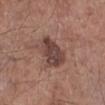  biopsy_status: not biopsied; imaged during a skin examination
  site: right lower leg
  image:
    source: total-body photography crop
    field_of_view_mm: 15
  patient:
    sex: male
    age_approx: 65